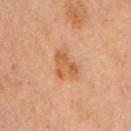Q: What are the patient's age and sex?
A: male, about 60 years old
Q: How was this image acquired?
A: ~15 mm crop, total-body skin-cancer survey
Q: Lesion location?
A: the upper back